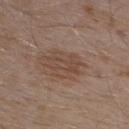- workup — catalogued during a skin exam; not biopsied
- image — ~15 mm crop, total-body skin-cancer survey
- lesion size — ≈4 mm
- anatomic site — the upper back
- subject — male, aged 53 to 57
- automated metrics — a footprint of about 6 mm²; an average lesion color of about L≈45 a*≈17 b*≈26 (CIELAB), about 7 CIELAB-L* units darker than the surrounding skin, and a normalized border contrast of about 5.5; an automated nevus-likeness rating near 0 out of 100
- illumination — white-light